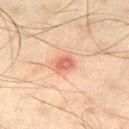acquisition: 15 mm crop, total-body photography | lighting: cross-polarized illumination | patient: male, in their mid-40s | automated metrics: a lesion color around L≈52 a*≈24 b*≈29 in CIELAB, roughly 10 lightness units darker than nearby skin, and a normalized lesion–skin contrast near 7; a classifier nevus-likeness of about 25/100 | diameter: about 2.5 mm | body site: the right thigh.